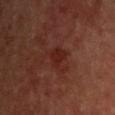Clinical impression:
No biopsy was performed on this lesion — it was imaged during a full skin examination and was not determined to be concerning.
Clinical summary:
A 15 mm crop from a total-body photograph taken for skin-cancer surveillance. Longest diameter approximately 2.5 mm. From the chest. A male subject, approximately 50 years of age.